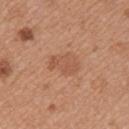Q: Was this lesion biopsied?
A: total-body-photography surveillance lesion; no biopsy
Q: How was this image acquired?
A: total-body-photography crop, ~15 mm field of view
Q: What is the anatomic site?
A: the left upper arm
Q: What are the patient's age and sex?
A: female, aged around 35